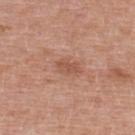Context: A female patient, aged 38 to 42. Approximately 3 mm at its widest. From the back. A 15 mm crop from a total-body photograph taken for skin-cancer surveillance.